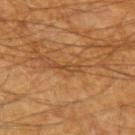workup: no biopsy performed (imaged during a skin exam) | illumination: cross-polarized | acquisition: ~15 mm crop, total-body skin-cancer survey | location: the left forearm | subject: male, aged around 60 | automated lesion analysis: a shape-asymmetry score of about 0.45 (0 = symmetric); an average lesion color of about L≈42 a*≈20 b*≈37 (CIELAB) and a lesion–skin lightness drop of about 7; border irregularity of about 6 on a 0–10 scale, a within-lesion color-variation index near 0/10, and a peripheral color-asymmetry measure near 0.